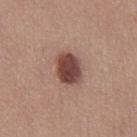Imaged during a routine full-body skin examination; the lesion was not biopsied and no histopathology is available. A male patient aged approximately 25. The recorded lesion diameter is about 4 mm. Imaged with white-light lighting. A 15 mm close-up tile from a total-body photography series done for melanoma screening. The lesion is on the chest.